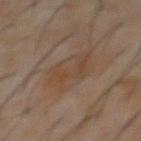The lesion is located on the abdomen.
The tile uses cross-polarized illumination.
Automated tile analysis of the lesion measured an area of roughly 13 mm² and two-axis asymmetry of about 0.3. And it measured a nevus-likeness score of about 0/100 and a detector confidence of about 100 out of 100 that the crop contains a lesion.
A male subject, roughly 60 years of age.
A close-up tile cropped from a whole-body skin photograph, about 15 mm across.
The lesion's longest dimension is about 4.5 mm.